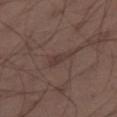The lesion was photographed on a routine skin check and not biopsied; there is no pathology result. Located on the left thigh. A male patient, roughly 40 years of age. A lesion tile, about 15 mm wide, cut from a 3D total-body photograph. The recorded lesion diameter is about 3.5 mm. Automated tile analysis of the lesion measured a footprint of about 4 mm², an outline eccentricity of about 0.9 (0 = round, 1 = elongated), and a symmetry-axis asymmetry near 0.4. The software also gave a lesion color around L≈36 a*≈15 b*≈19 in CIELAB, a lesion–skin lightness drop of about 6, and a lesion-to-skin contrast of about 5.5 (normalized; higher = more distinct). And it measured a border-irregularity rating of about 4/10, a color-variation rating of about 1.5/10, and radial color variation of about 0.5.